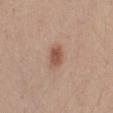Findings:
– notes · total-body-photography surveillance lesion; no biopsy
– tile lighting · white-light illumination
– anatomic site · the mid back
– patient · male, roughly 30 years of age
– diameter · about 3 mm
– acquisition · ~15 mm crop, total-body skin-cancer survey